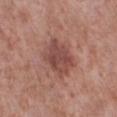{
  "biopsy_status": "not biopsied; imaged during a skin examination",
  "lighting": "white-light",
  "image": {
    "source": "total-body photography crop",
    "field_of_view_mm": 15
  },
  "lesion_size": {
    "long_diameter_mm_approx": 4.5
  },
  "patient": {
    "sex": "male",
    "age_approx": 55
  },
  "site": "right lower leg"
}